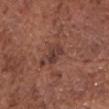Assessment: This lesion was catalogued during total-body skin photography and was not selected for biopsy. Acquisition and patient details: A male subject, aged approximately 75. An algorithmic analysis of the crop reported a lesion area of about 4.5 mm², an outline eccentricity of about 0.8 (0 = round, 1 = elongated), and a symmetry-axis asymmetry near 0.2. The analysis additionally found border irregularity of about 2.5 on a 0–10 scale, a within-lesion color-variation index near 3.5/10, and radial color variation of about 1.5. The analysis additionally found a classifier nevus-likeness of about 0/100 and lesion-presence confidence of about 95/100. The tile uses white-light illumination. On the chest. A lesion tile, about 15 mm wide, cut from a 3D total-body photograph. The recorded lesion diameter is about 3 mm.